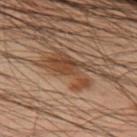No biopsy was performed on this lesion — it was imaged during a full skin examination and was not determined to be concerning. On the arm. About 5.5 mm across. A 15 mm close-up extracted from a 3D total-body photography capture. Captured under cross-polarized illumination. The subject is a male aged approximately 40.